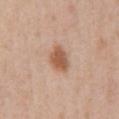Recorded during total-body skin imaging; not selected for excision or biopsy. Located on the front of the torso. A male patient aged approximately 55. A 15 mm close-up extracted from a 3D total-body photography capture. Imaged with white-light lighting. The total-body-photography lesion software estimated an average lesion color of about L≈56 a*≈21 b*≈32 (CIELAB), a lesion–skin lightness drop of about 12, and a normalized lesion–skin contrast near 8.5.